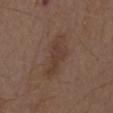<tbp_lesion>
  <biopsy_status>not biopsied; imaged during a skin examination</biopsy_status>
  <image>
    <source>total-body photography crop</source>
    <field_of_view_mm>15</field_of_view_mm>
  </image>
  <site>mid back</site>
  <patient>
    <sex>male</sex>
    <age_approx>70</age_approx>
  </patient>
</tbp_lesion>